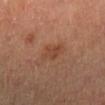follow-up=catalogued during a skin exam; not biopsied
location=the left lower leg
illumination=cross-polarized illumination
image=15 mm crop, total-body photography
subject=male, aged around 65